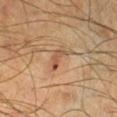Captured during whole-body skin photography for melanoma surveillance; the lesion was not biopsied. The lesion is on the right lower leg. Approximately 3 mm at its widest. The tile uses cross-polarized illumination. A male subject, aged approximately 45. Cropped from a whole-body photographic skin survey; the tile spans about 15 mm.